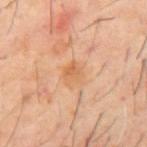Recorded during total-body skin imaging; not selected for excision or biopsy.
The lesion is located on the mid back.
A 15 mm close-up tile from a total-body photography series done for melanoma screening.
Imaged with cross-polarized lighting.
A male subject, aged 58–62.